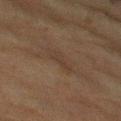biopsy status: catalogued during a skin exam; not biopsied | acquisition: 15 mm crop, total-body photography | patient: male, about 75 years old | illumination: cross-polarized | lesion diameter: about 3.5 mm | body site: the right upper arm | automated lesion analysis: a detector confidence of about 65 out of 100 that the crop contains a lesion.